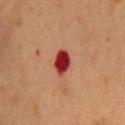Findings:
– biopsy status: total-body-photography surveillance lesion; no biopsy
– lesion diameter: ~3.5 mm (longest diameter)
– patient: female, aged approximately 40
– lighting: cross-polarized illumination
– location: the chest
– imaging modality: ~15 mm tile from a whole-body skin photo